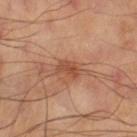follow-up = imaged on a skin check; not biopsied | lesion size = ≈2.5 mm | acquisition = 15 mm crop, total-body photography | anatomic site = the right thigh | lighting = cross-polarized.